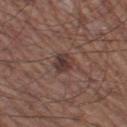Assessment:
Recorded during total-body skin imaging; not selected for excision or biopsy.
Image and clinical context:
A male patient, aged approximately 60. The lesion-visualizer software estimated an average lesion color of about L≈36 a*≈17 b*≈20 (CIELAB), roughly 10 lightness units darker than nearby skin, and a lesion-to-skin contrast of about 8.5 (normalized; higher = more distinct). It also reported border irregularity of about 2 on a 0–10 scale, a color-variation rating of about 3.5/10, and peripheral color asymmetry of about 1. Captured under white-light illumination. Measured at roughly 3 mm in maximum diameter. From the leg. Cropped from a whole-body photographic skin survey; the tile spans about 15 mm.